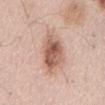patient = male, aged 53–57 | diameter = ~6.5 mm (longest diameter) | image source = total-body-photography crop, ~15 mm field of view | tile lighting = white-light illumination | body site = the mid back.